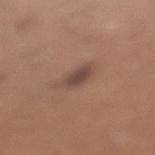Assessment: This lesion was catalogued during total-body skin photography and was not selected for biopsy. Image and clinical context: This is a white-light tile. The lesion is located on the left lower leg. Cropped from a whole-body photographic skin survey; the tile spans about 15 mm. A male subject approximately 40 years of age.